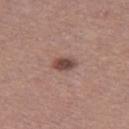notes = total-body-photography surveillance lesion; no biopsy | image source = 15 mm crop, total-body photography | subject = female, in their mid- to late 30s | body site = the leg | lighting = white-light illumination | lesion diameter = ≈3 mm.